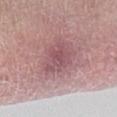The lesion was photographed on a routine skin check and not biopsied; there is no pathology result.
Measured at roughly 4 mm in maximum diameter.
This image is a 15 mm lesion crop taken from a total-body photograph.
The tile uses white-light illumination.
The lesion is located on the right lower leg.
A male patient approximately 60 years of age.